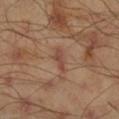notes: no biopsy performed (imaged during a skin exam) | image: ~15 mm crop, total-body skin-cancer survey | location: the right lower leg | subject: male, in their mid-40s | automated lesion analysis: a shape eccentricity near 0.95 and a shape-asymmetry score of about 0.35 (0 = symmetric); a lesion color around L≈44 a*≈22 b*≈26 in CIELAB, roughly 8 lightness units darker than nearby skin, and a normalized lesion–skin contrast near 6.5; a border-irregularity rating of about 4/10 and peripheral color asymmetry of about 0 | lesion size: about 3 mm.